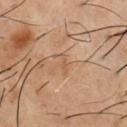Part of a total-body skin-imaging series; this lesion was reviewed on a skin check and was not flagged for biopsy. The tile uses cross-polarized illumination. From the chest. A male patient aged 53–57. A region of skin cropped from a whole-body photographic capture, roughly 15 mm wide.